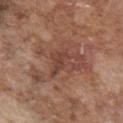Findings:
– notes: no biopsy performed (imaged during a skin exam)
– size: ≈7.5 mm
– site: the chest
– subject: male, aged 73–77
– TBP lesion metrics: an average lesion color of about L≈45 a*≈21 b*≈26 (CIELAB) and a normalized lesion–skin contrast near 6; a classifier nevus-likeness of about 0/100 and a lesion-detection confidence of about 75/100
– image: ~15 mm crop, total-body skin-cancer survey
– illumination: white-light